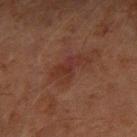Case summary:
• follow-up · no biopsy performed (imaged during a skin exam)
• subject · male, in their 60s
• diameter · about 4.5 mm
• automated metrics · a footprint of about 7 mm² and a shape-asymmetry score of about 0.45 (0 = symmetric); an average lesion color of about L≈26 a*≈20 b*≈22 (CIELAB), about 5 CIELAB-L* units darker than the surrounding skin, and a normalized border contrast of about 6; border irregularity of about 5.5 on a 0–10 scale and a color-variation rating of about 3/10; a nevus-likeness score of about 10/100 and a lesion-detection confidence of about 100/100
• body site · the left forearm
• image source · total-body-photography crop, ~15 mm field of view